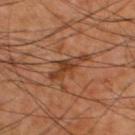Q: What did automated image analysis measure?
A: a lesion color around L≈40 a*≈22 b*≈33 in CIELAB, a lesion–skin lightness drop of about 9, and a lesion-to-skin contrast of about 7.5 (normalized; higher = more distinct)
Q: What lighting was used for the tile?
A: cross-polarized illumination
Q: What is the imaging modality?
A: 15 mm crop, total-body photography
Q: Who is the patient?
A: male, in their 60s
Q: How large is the lesion?
A: about 5.5 mm
Q: What is the anatomic site?
A: the upper back